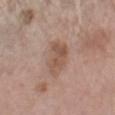biopsy status: imaged on a skin check; not biopsied
imaging modality: total-body-photography crop, ~15 mm field of view
site: the right forearm
diameter: about 5 mm
patient: female, roughly 70 years of age
lighting: white-light illumination
TBP lesion metrics: a shape eccentricity near 0.85 and a symmetry-axis asymmetry near 0.2; a border-irregularity index near 3/10 and radial color variation of about 1.5; a nevus-likeness score of about 0/100